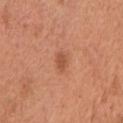{"biopsy_status": "not biopsied; imaged during a skin examination", "site": "chest", "patient": {"sex": "male", "age_approx": 65}, "lesion_size": {"long_diameter_mm_approx": 2.5}, "image": {"source": "total-body photography crop", "field_of_view_mm": 15}, "lighting": "white-light"}